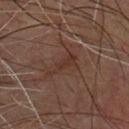{
  "biopsy_status": "not biopsied; imaged during a skin examination",
  "lesion_size": {
    "long_diameter_mm_approx": 4.0
  },
  "image": {
    "source": "total-body photography crop",
    "field_of_view_mm": 15
  },
  "site": "chest",
  "lighting": "cross-polarized",
  "patient": {
    "sex": "male",
    "age_approx": 55
  }
}